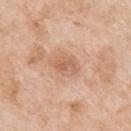follow-up=no biopsy performed (imaged during a skin exam) | site=the arm | lesion diameter=about 3.5 mm | patient=female, about 75 years old | acquisition=~15 mm crop, total-body skin-cancer survey.